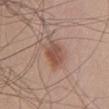<case>
  <biopsy_status>not biopsied; imaged during a skin examination</biopsy_status>
  <lesion_size>
    <long_diameter_mm_approx>4.5</long_diameter_mm_approx>
  </lesion_size>
  <lighting>white-light</lighting>
  <site>chest</site>
  <patient>
    <sex>male</sex>
    <age_approx>55</age_approx>
  </patient>
  <image>
    <source>total-body photography crop</source>
    <field_of_view_mm>15</field_of_view_mm>
  </image>
</case>